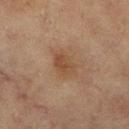follow-up = imaged on a skin check; not biopsied | image = 15 mm crop, total-body photography | anatomic site = the right lower leg | tile lighting = cross-polarized | patient = female, about 80 years old | automated metrics = a footprint of about 5 mm² and two-axis asymmetry of about 0.2; a border-irregularity index near 2.5/10 and a within-lesion color-variation index near 2/10; a nevus-likeness score of about 45/100 and a lesion-detection confidence of about 100/100.